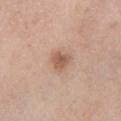biopsy status = no biopsy performed (imaged during a skin exam) | automated metrics = a border-irregularity rating of about 2.5/10 and internal color variation of about 2.5 on a 0–10 scale; a classifier nevus-likeness of about 70/100 | anatomic site = the left lower leg | acquisition = ~15 mm tile from a whole-body skin photo | illumination = white-light | patient = female, in their mid-50s | lesion size = ≈2.5 mm.